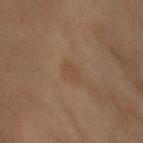Q: What is the anatomic site?
A: the left upper arm
Q: How was this image acquired?
A: 15 mm crop, total-body photography
Q: Who is the patient?
A: female, aged 53–57